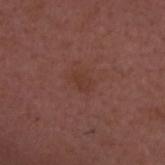{"lighting": "white-light", "image": {"source": "total-body photography crop", "field_of_view_mm": 15}, "patient": {"sex": "male", "age_approx": 50}, "site": "head or neck"}